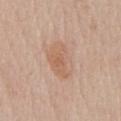follow-up: total-body-photography surveillance lesion; no biopsy
size: ≈5 mm
lighting: white-light illumination
subject: male, aged 63 to 67
image-analysis metrics: an average lesion color of about L≈61 a*≈19 b*≈31 (CIELAB), a lesion–skin lightness drop of about 7, and a normalized lesion–skin contrast near 5.5; border irregularity of about 2.5 on a 0–10 scale, internal color variation of about 3.5 on a 0–10 scale, and radial color variation of about 1; a classifier nevus-likeness of about 25/100
imaging modality: ~15 mm crop, total-body skin-cancer survey
location: the front of the torso